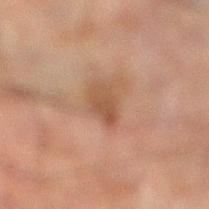Imaged during a routine full-body skin examination; the lesion was not biopsied and no histopathology is available.
A male subject aged around 60.
This image is a 15 mm lesion crop taken from a total-body photograph.
Captured under cross-polarized illumination.
The lesion is on the leg.